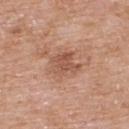Findings:
• notes — imaged on a skin check; not biopsied
• image source — 15 mm crop, total-body photography
• image-analysis metrics — an outline eccentricity of about 0.65 (0 = round, 1 = elongated); roughly 9 lightness units darker than nearby skin and a normalized lesion–skin contrast near 6.5; a classifier nevus-likeness of about 0/100 and a lesion-detection confidence of about 100/100
• illumination — white-light illumination
• location — the upper back
• subject — male, about 75 years old
• lesion size — ≈3.5 mm